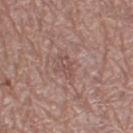Q: Was a biopsy performed?
A: no biopsy performed (imaged during a skin exam)
Q: How large is the lesion?
A: ≈3 mm
Q: How was the tile lit?
A: white-light illumination
Q: What did automated image analysis measure?
A: a shape eccentricity near 0.9 and two-axis asymmetry of about 0.3; a mean CIELAB color near L≈50 a*≈19 b*≈23 and a lesion-to-skin contrast of about 5 (normalized; higher = more distinct); a border-irregularity index near 4/10, a color-variation rating of about 1/10, and peripheral color asymmetry of about 0; a nevus-likeness score of about 0/100 and a lesion-detection confidence of about 95/100
Q: Patient demographics?
A: female, aged approximately 60
Q: What is the anatomic site?
A: the right thigh
Q: What is the imaging modality?
A: ~15 mm tile from a whole-body skin photo